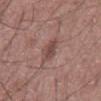On the chest. A male patient, approximately 60 years of age. A 15 mm crop from a total-body photograph taken for skin-cancer surveillance. Approximately 3.5 mm at its widest.